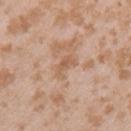Assessment:
No biopsy was performed on this lesion — it was imaged during a full skin examination and was not determined to be concerning.
Clinical summary:
A region of skin cropped from a whole-body photographic capture, roughly 15 mm wide. A female subject in their mid- to late 20s. Located on the arm.